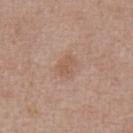No biopsy was performed on this lesion — it was imaged during a full skin examination and was not determined to be concerning.
This image is a 15 mm lesion crop taken from a total-body photograph.
On the abdomen.
The patient is a male in their 50s.